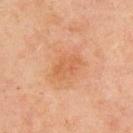Assessment: The lesion was photographed on a routine skin check and not biopsied; there is no pathology result. Acquisition and patient details: Located on the upper back. A 15 mm crop from a total-body photograph taken for skin-cancer surveillance. The subject is a male roughly 45 years of age.